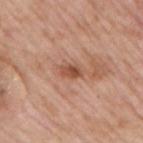follow-up: catalogued during a skin exam; not biopsied | acquisition: ~15 mm tile from a whole-body skin photo | TBP lesion metrics: a lesion-detection confidence of about 100/100 | site: the right upper arm | lighting: white-light illumination | subject: male, aged 73 to 77.